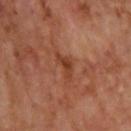biopsy_status: not biopsied; imaged during a skin examination
site: upper back
patient:
  sex: male
  age_approx: 70
image:
  source: total-body photography crop
  field_of_view_mm: 15
lighting: cross-polarized
lesion_size:
  long_diameter_mm_approx: 3.5